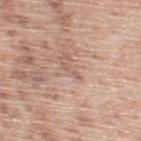lighting: white-light
automated lesion analysis: a lesion area of about 1.5 mm² and an outline eccentricity of about 0.95 (0 = round, 1 = elongated); a border-irregularity rating of about 5.5/10, a color-variation rating of about 0/10, and a peripheral color-asymmetry measure near 0
imaging modality: ~15 mm tile from a whole-body skin photo
lesion size: about 2.5 mm
patient: male, about 55 years old
anatomic site: the upper back